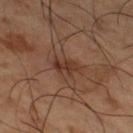workup = imaged on a skin check; not biopsied